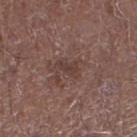The lesion was tiled from a total-body skin photograph and was not biopsied.
From the left lower leg.
A 15 mm crop from a total-body photograph taken for skin-cancer surveillance.
Approximately 3 mm at its widest.
The patient is a male in their mid-70s.
Automated image analysis of the tile measured a footprint of about 4 mm² and a symmetry-axis asymmetry near 0.4. It also reported a lesion–skin lightness drop of about 7 and a normalized lesion–skin contrast near 6. It also reported a border-irregularity rating of about 4.5/10, a within-lesion color-variation index near 1/10, and peripheral color asymmetry of about 0.5.